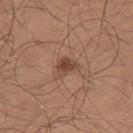| field | value |
|---|---|
| imaging modality | ~15 mm tile from a whole-body skin photo |
| body site | the left upper arm |
| patient | male, about 25 years old |